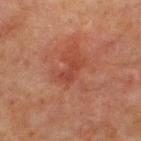Part of a total-body skin-imaging series; this lesion was reviewed on a skin check and was not flagged for biopsy. A male patient, in their mid-60s. An algorithmic analysis of the crop reported an average lesion color of about L≈35 a*≈25 b*≈26 (CIELAB) and a lesion–skin lightness drop of about 5. On the chest. The recorded lesion diameter is about 3 mm. The tile uses cross-polarized illumination. A 15 mm close-up tile from a total-body photography series done for melanoma screening.